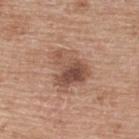Clinical impression: The lesion was photographed on a routine skin check and not biopsied; there is no pathology result.